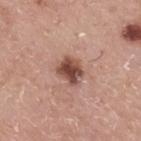Recorded during total-body skin imaging; not selected for excision or biopsy.
The lesion is on the mid back.
A male subject aged around 75.
Automated image analysis of the tile measured a lesion area of about 7.5 mm², an outline eccentricity of about 0.55 (0 = round, 1 = elongated), and a shape-asymmetry score of about 0.25 (0 = symmetric). The analysis additionally found an average lesion color of about L≈48 a*≈22 b*≈26 (CIELAB) and a normalized lesion–skin contrast near 10.5. The software also gave a border-irregularity index near 2/10. The software also gave a classifier nevus-likeness of about 90/100.
The tile uses white-light illumination.
A 15 mm close-up tile from a total-body photography series done for melanoma screening.
The recorded lesion diameter is about 3 mm.